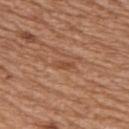biopsy status: total-body-photography surveillance lesion; no biopsy
subject: male, in their mid- to late 60s
body site: the upper back
image source: total-body-photography crop, ~15 mm field of view